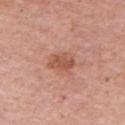Q: Was this lesion biopsied?
A: no biopsy performed (imaged during a skin exam)
Q: Patient demographics?
A: female, roughly 65 years of age
Q: Where on the body is the lesion?
A: the arm
Q: What is the imaging modality?
A: total-body-photography crop, ~15 mm field of view